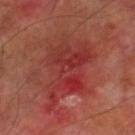The tile uses cross-polarized illumination. A male patient, aged 68–72. Automated tile analysis of the lesion measured a lesion area of about 32 mm², an outline eccentricity of about 0.8 (0 = round, 1 = elongated), and two-axis asymmetry of about 0.4. The lesion is located on the right lower leg. Measured at roughly 9.5 mm in maximum diameter. This image is a 15 mm lesion crop taken from a total-body photograph.